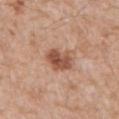Notes:
• body site: the back
• lesion diameter: about 3.5 mm
• patient: male, aged 58 to 62
• tile lighting: white-light illumination
• acquisition: 15 mm crop, total-body photography